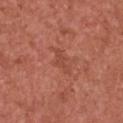workup — no biopsy performed (imaged during a skin exam)
body site — the chest
size — about 3.5 mm
image — total-body-photography crop, ~15 mm field of view
subject — female, aged 48–52
tile lighting — white-light